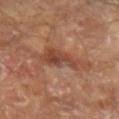Imaged with cross-polarized lighting.
Located on the left forearm.
A region of skin cropped from a whole-body photographic capture, roughly 15 mm wide.
A male patient, in their mid-60s.